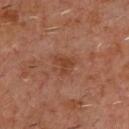biopsy status: imaged on a skin check; not biopsied
size: about 2.5 mm
patient: male, aged around 60
image source: ~15 mm crop, total-body skin-cancer survey
body site: the chest